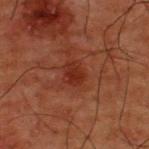The lesion was tiled from a total-body skin photograph and was not biopsied. The patient is a male aged around 50. From the upper back. Cropped from a total-body skin-imaging series; the visible field is about 15 mm.